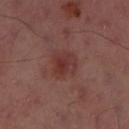| field | value |
|---|---|
| workup | imaged on a skin check; not biopsied |
| location | the left thigh |
| imaging modality | 15 mm crop, total-body photography |